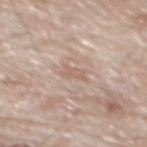notes: imaged on a skin check; not biopsied
location: the back
patient: male, aged 78 to 82
imaging modality: 15 mm crop, total-body photography